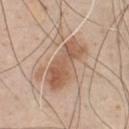<case>
<biopsy_status>not biopsied; imaged during a skin examination</biopsy_status>
<automated_metrics>
  <area_mm2_approx>15.0</area_mm2_approx>
  <eccentricity>0.9</eccentricity>
  <shape_asymmetry>0.25</shape_asymmetry>
  <cielab_L>57</cielab_L>
  <cielab_a>19</cielab_a>
  <cielab_b>30</cielab_b>
  <vs_skin_darker_L>11.0</vs_skin_darker_L>
  <vs_skin_contrast_norm>8.0</vs_skin_contrast_norm>
</automated_metrics>
<lighting>white-light</lighting>
<lesion_size>
  <long_diameter_mm_approx>6.5</long_diameter_mm_approx>
</lesion_size>
<site>front of the torso</site>
<patient>
  <sex>male</sex>
  <age_approx>45</age_approx>
</patient>
<image>
  <source>total-body photography crop</source>
  <field_of_view_mm>15</field_of_view_mm>
</image>
</case>